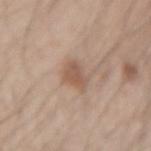Context:
This is a white-light tile. Measured at roughly 3 mm in maximum diameter. A roughly 15 mm field-of-view crop from a total-body skin photograph. A male subject approximately 30 years of age. From the left forearm.